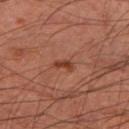Part of a total-body skin-imaging series; this lesion was reviewed on a skin check and was not flagged for biopsy.
A 15 mm close-up tile from a total-body photography series done for melanoma screening.
A male subject, in their 60s.
The lesion is on the left thigh.
This is a cross-polarized tile.